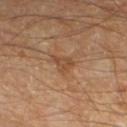Clinical impression: The lesion was photographed on a routine skin check and not biopsied; there is no pathology result. Acquisition and patient details: Captured under cross-polarized illumination. Cropped from a whole-body photographic skin survey; the tile spans about 15 mm. From the left lower leg. Automated image analysis of the tile measured a border-irregularity index near 4/10. The patient is aged 53–57.